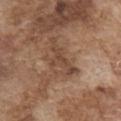Imaged during a routine full-body skin examination; the lesion was not biopsied and no histopathology is available. The lesion is located on the chest. A male subject, aged 73 to 77. The recorded lesion diameter is about 5.5 mm. A roughly 15 mm field-of-view crop from a total-body skin photograph. Captured under white-light illumination.